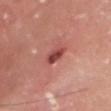Part of a total-body skin-imaging series; this lesion was reviewed on a skin check and was not flagged for biopsy. Located on the head or neck. A close-up tile cropped from a whole-body skin photograph, about 15 mm across. Imaged with white-light lighting. The patient is a male roughly 65 years of age. Automated image analysis of the tile measured an area of roughly 4 mm² and two-axis asymmetry of about 0.25. It also reported a lesion color around L≈46 a*≈31 b*≈26 in CIELAB, roughly 13 lightness units darker than nearby skin, and a normalized border contrast of about 9. Measured at roughly 2.5 mm in maximum diameter.